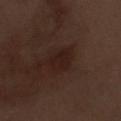Image and clinical context: From the left forearm. A male subject, roughly 70 years of age. Cropped from a total-body skin-imaging series; the visible field is about 15 mm.